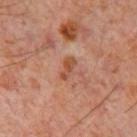image: 15 mm crop, total-body photography
lesion diameter: ≈3 mm
body site: the chest
subject: male, aged 58–62
TBP lesion metrics: an area of roughly 3 mm² and a shape-asymmetry score of about 0.35 (0 = symmetric); a lesion color around L≈46 a*≈24 b*≈32 in CIELAB, roughly 8 lightness units darker than nearby skin, and a normalized lesion–skin contrast near 7.5; a classifier nevus-likeness of about 0/100 and a lesion-detection confidence of about 100/100